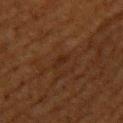{"biopsy_status": "not biopsied; imaged during a skin examination", "lesion_size": {"long_diameter_mm_approx": 2.5}, "lighting": "cross-polarized", "site": "back", "automated_metrics": {"area_mm2_approx": 2.5, "eccentricity": 0.9, "shape_asymmetry": 0.35, "cielab_L": 20, "cielab_a": 17, "cielab_b": 24, "vs_skin_darker_L": 4.0, "vs_skin_contrast_norm": 5.5, "border_irregularity_0_10": 3.5, "color_variation_0_10": 0.0, "peripheral_color_asymmetry": 0.0}, "image": {"source": "total-body photography crop", "field_of_view_mm": 15}, "patient": {"sex": "female", "age_approx": 40}}